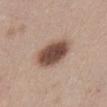Part of a total-body skin-imaging series; this lesion was reviewed on a skin check and was not flagged for biopsy.
A female patient about 40 years old.
Automated tile analysis of the lesion measured roughly 18 lightness units darker than nearby skin and a normalized lesion–skin contrast near 12. It also reported a border-irregularity rating of about 1/10 and a within-lesion color-variation index near 4.5/10. And it measured a classifier nevus-likeness of about 95/100 and a detector confidence of about 100 out of 100 that the crop contains a lesion.
Measured at roughly 4.5 mm in maximum diameter.
On the front of the torso.
A close-up tile cropped from a whole-body skin photograph, about 15 mm across.